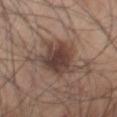Assessment: Captured during whole-body skin photography for melanoma surveillance; the lesion was not biopsied. Image and clinical context: Located on the chest. Approximately 7 mm at its widest. A male patient aged around 55. Imaged with white-light lighting. A 15 mm close-up extracted from a 3D total-body photography capture.